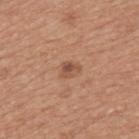Part of a total-body skin-imaging series; this lesion was reviewed on a skin check and was not flagged for biopsy. Cropped from a whole-body photographic skin survey; the tile spans about 15 mm. The tile uses white-light illumination. The subject is a male approximately 65 years of age. The lesion is located on the back. Measured at roughly 2.5 mm in maximum diameter.